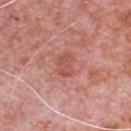<case>
  <patient>
    <sex>male</sex>
    <age_approx>80</age_approx>
  </patient>
  <image>
    <source>total-body photography crop</source>
    <field_of_view_mm>15</field_of_view_mm>
  </image>
  <lesion_size>
    <long_diameter_mm_approx>3.0</long_diameter_mm_approx>
  </lesion_size>
  <automated_metrics>
    <area_mm2_approx>4.5</area_mm2_approx>
    <eccentricity>0.8</eccentricity>
    <shape_asymmetry>0.3</shape_asymmetry>
    <border_irregularity_0_10>3.0</border_irregularity_0_10>
    <color_variation_0_10>2.0</color_variation_0_10>
    <peripheral_color_asymmetry>0.5</peripheral_color_asymmetry>
    <nevus_likeness_0_100>0</nevus_likeness_0_100>
    <lesion_detection_confidence_0_100>100</lesion_detection_confidence_0_100>
  </automated_metrics>
  <site>front of the torso</site>
  <lighting>white-light</lighting>
</case>